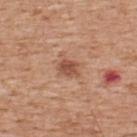| key | value |
|---|---|
| workup | catalogued during a skin exam; not biopsied |
| image | 15 mm crop, total-body photography |
| patient | male, aged around 60 |
| tile lighting | white-light illumination |
| lesion diameter | about 3 mm |
| body site | the back |